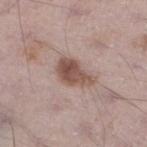  biopsy_status: not biopsied; imaged during a skin examination
  image:
    source: total-body photography crop
    field_of_view_mm: 15
  automated_metrics:
    border_irregularity_0_10: 4.0
    color_variation_0_10: 4.5
    peripheral_color_asymmetry: 2.0
    nevus_likeness_0_100: 85
    lesion_detection_confidence_0_100: 100
  lesion_size:
    long_diameter_mm_approx: 4.5
  lighting: white-light
  site: left lower leg
  patient:
    sex: male
    age_approx: 70